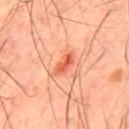Image and clinical context: Longest diameter approximately 3.5 mm. A male subject about 45 years old. Captured under cross-polarized illumination. A region of skin cropped from a whole-body photographic capture, roughly 15 mm wide. An algorithmic analysis of the crop reported a border-irregularity rating of about 2.5/10, a color-variation rating of about 4.5/10, and radial color variation of about 1.5. The lesion is located on the back.